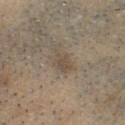Notes:
– notes: no biopsy performed (imaged during a skin exam)
– image: 15 mm crop, total-body photography
– diameter: ~2.5 mm (longest diameter)
– image-analysis metrics: a mean CIELAB color near L≈48 a*≈10 b*≈25, a lesion–skin lightness drop of about 7, and a normalized border contrast of about 5.5; a border-irregularity index near 2/10, a color-variation rating of about 2.5/10, and a peripheral color-asymmetry measure near 1; an automated nevus-likeness rating near 10 out of 100 and a detector confidence of about 65 out of 100 that the crop contains a lesion
– patient: male, aged approximately 40
– illumination: white-light illumination
– site: the head or neck